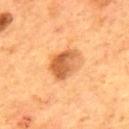Context: A 15 mm crop from a total-body photograph taken for skin-cancer surveillance. Captured under cross-polarized illumination. Located on the mid back. Measured at roughly 4 mm in maximum diameter. A male subject, in their mid-50s.